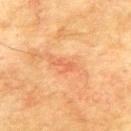Q: Was a biopsy performed?
A: no biopsy performed (imaged during a skin exam)
Q: What lighting was used for the tile?
A: cross-polarized illumination
Q: Lesion location?
A: the upper back
Q: Who is the patient?
A: male, roughly 75 years of age
Q: What is the lesion's diameter?
A: ≈2.5 mm
Q: How was this image acquired?
A: ~15 mm crop, total-body skin-cancer survey
Q: What did automated image analysis measure?
A: a border-irregularity index near 6/10 and a within-lesion color-variation index near 0/10; a nevus-likeness score of about 0/100 and a lesion-detection confidence of about 100/100